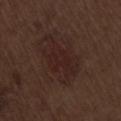No biopsy was performed on this lesion — it was imaged during a full skin examination and was not determined to be concerning.
Measured at roughly 7 mm in maximum diameter.
This image is a 15 mm lesion crop taken from a total-body photograph.
The lesion-visualizer software estimated a footprint of about 18 mm², a shape eccentricity near 0.8, and a symmetry-axis asymmetry near 0.35. The software also gave a mean CIELAB color near L≈22 a*≈17 b*≈18, a lesion–skin lightness drop of about 5, and a normalized border contrast of about 6.5. The software also gave internal color variation of about 2.5 on a 0–10 scale.
A male patient in their 70s.
Located on the lower back.
Imaged with white-light lighting.